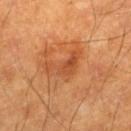Clinical impression: Recorded during total-body skin imaging; not selected for excision or biopsy. Context: A male patient, aged around 60. From the leg. The lesion-visualizer software estimated a mean CIELAB color near L≈47 a*≈27 b*≈38 and a normalized border contrast of about 6. It also reported a classifier nevus-likeness of about 60/100. Imaged with cross-polarized lighting. Approximately 5 mm at its widest. A close-up tile cropped from a whole-body skin photograph, about 15 mm across.